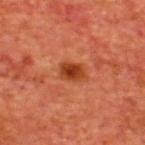<record>
<biopsy_status>not biopsied; imaged during a skin examination</biopsy_status>
<patient>
  <sex>male</sex>
  <age_approx>65</age_approx>
</patient>
<image>
  <source>total-body photography crop</source>
  <field_of_view_mm>15</field_of_view_mm>
</image>
<lighting>cross-polarized</lighting>
<automated_metrics>
  <cielab_L>38</cielab_L>
  <cielab_a>30</cielab_a>
  <cielab_b>37</cielab_b>
  <vs_skin_darker_L>11.0</vs_skin_darker_L>
  <vs_skin_contrast_norm>9.0</vs_skin_contrast_norm>
  <border_irregularity_0_10>2.0</border_irregularity_0_10>
  <color_variation_0_10>4.0</color_variation_0_10>
  <nevus_likeness_0_100>100</nevus_likeness_0_100>
  <lesion_detection_confidence_0_100>100</lesion_detection_confidence_0_100>
</automated_metrics>
<site>upper back</site>
</record>